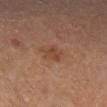{
  "patient": {
    "sex": "male",
    "age_approx": 65
  },
  "automated_metrics": {
    "shape_asymmetry": 0.3,
    "cielab_L": 39,
    "cielab_a": 19,
    "cielab_b": 28,
    "vs_skin_contrast_norm": 5.5
  },
  "image": {
    "source": "total-body photography crop",
    "field_of_view_mm": 15
  },
  "lighting": "cross-polarized",
  "site": "left lower leg",
  "lesion_size": {
    "long_diameter_mm_approx": 2.5
  }
}